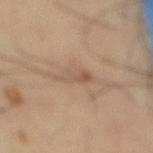Acquisition and patient details: Captured under cross-polarized illumination. From the lower back. A male subject, aged around 40. A roughly 15 mm field-of-view crop from a total-body skin photograph. Automated tile analysis of the lesion measured a lesion color around L≈53 a*≈16 b*≈28 in CIELAB, a lesion–skin lightness drop of about 8, and a lesion-to-skin contrast of about 5.5 (normalized; higher = more distinct). The lesion's longest dimension is about 4 mm.